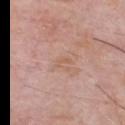Clinical impression:
Part of a total-body skin-imaging series; this lesion was reviewed on a skin check and was not flagged for biopsy.
Context:
The subject is a male about 60 years old. The lesion is on the chest. A close-up tile cropped from a whole-body skin photograph, about 15 mm across. The lesion's longest dimension is about 3 mm. The tile uses white-light illumination.